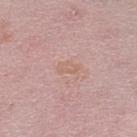Q: Was this lesion biopsied?
A: imaged on a skin check; not biopsied
Q: What kind of image is this?
A: total-body-photography crop, ~15 mm field of view
Q: Illumination type?
A: white-light
Q: What are the patient's age and sex?
A: female, aged around 45
Q: Lesion size?
A: ~2.5 mm (longest diameter)
Q: Lesion location?
A: the left lower leg